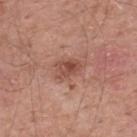Assessment:
Part of a total-body skin-imaging series; this lesion was reviewed on a skin check and was not flagged for biopsy.
Image and clinical context:
A male patient, in their mid- to late 50s. From the mid back. A 15 mm close-up tile from a total-body photography series done for melanoma screening. The lesion's longest dimension is about 4 mm. The tile uses white-light illumination. Automated image analysis of the tile measured a lesion color around L≈50 a*≈23 b*≈28 in CIELAB, roughly 10 lightness units darker than nearby skin, and a normalized border contrast of about 7. It also reported border irregularity of about 3 on a 0–10 scale, a color-variation rating of about 5/10, and peripheral color asymmetry of about 2. It also reported an automated nevus-likeness rating near 20 out of 100 and a lesion-detection confidence of about 100/100.